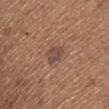Clinical impression: Part of a total-body skin-imaging series; this lesion was reviewed on a skin check and was not flagged for biopsy. Acquisition and patient details: A close-up tile cropped from a whole-body skin photograph, about 15 mm across. The total-body-photography lesion software estimated an average lesion color of about L≈46 a*≈20 b*≈25 (CIELAB), a lesion–skin lightness drop of about 8, and a lesion-to-skin contrast of about 7 (normalized; higher = more distinct). The software also gave a border-irregularity index near 3/10, internal color variation of about 2.5 on a 0–10 scale, and peripheral color asymmetry of about 1. Captured under white-light illumination. A male subject aged 63–67. The lesion is located on the upper back. Longest diameter approximately 3 mm.